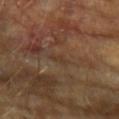follow-up: imaged on a skin check; not biopsied
lesion diameter: about 2.5 mm
illumination: cross-polarized illumination
subject: male, approximately 70 years of age
image source: total-body-photography crop, ~15 mm field of view
automated lesion analysis: an area of roughly 2.5 mm², a shape eccentricity near 0.85, and two-axis asymmetry of about 0.25
body site: the arm